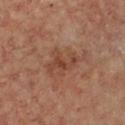Q: What kind of image is this?
A: ~15 mm tile from a whole-body skin photo
Q: How was the tile lit?
A: cross-polarized illumination
Q: Where on the body is the lesion?
A: the chest
Q: Who is the patient?
A: female, aged around 45